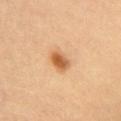No biopsy was performed on this lesion — it was imaged during a full skin examination and was not determined to be concerning. The lesion's longest dimension is about 3 mm. A 15 mm close-up extracted from a 3D total-body photography capture. Captured under cross-polarized illumination. The lesion-visualizer software estimated a shape eccentricity near 0.7 and a symmetry-axis asymmetry near 0.15. It also reported a lesion–skin lightness drop of about 13. The analysis additionally found a border-irregularity rating of about 1.5/10, a color-variation rating of about 4/10, and peripheral color asymmetry of about 1. The subject is a male aged around 20. On the chest.